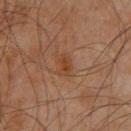Clinical impression:
Captured during whole-body skin photography for melanoma surveillance; the lesion was not biopsied.